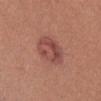No biopsy was performed on this lesion — it was imaged during a full skin examination and was not determined to be concerning. The patient is a female in their mid- to late 30s. Captured under white-light illumination. Approximately 4 mm at its widest. The lesion is located on the lower back. A 15 mm crop from a total-body photograph taken for skin-cancer surveillance.